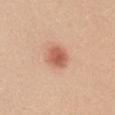<lesion>
<biopsy_status>not biopsied; imaged during a skin examination</biopsy_status>
<site>mid back</site>
<automated_metrics>
  <border_irregularity_0_10>1.0</border_irregularity_0_10>
  <color_variation_0_10>3.0</color_variation_0_10>
  <peripheral_color_asymmetry>1.0</peripheral_color_asymmetry>
</automated_metrics>
<image>
  <source>total-body photography crop</source>
  <field_of_view_mm>15</field_of_view_mm>
</image>
<lighting>white-light</lighting>
<patient>
  <sex>female</sex>
  <age_approx>20</age_approx>
</patient>
</lesion>